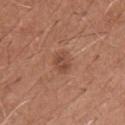Q: What are the patient's age and sex?
A: male, aged 63 to 67
Q: How was this image acquired?
A: ~15 mm crop, total-body skin-cancer survey
Q: Where on the body is the lesion?
A: the chest